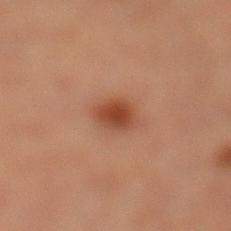The subject is a male aged around 60. This is a cross-polarized tile. A 15 mm close-up extracted from a 3D total-body photography capture. Automated tile analysis of the lesion measured a lesion–skin lightness drop of about 9 and a normalized lesion–skin contrast near 9. It also reported a border-irregularity index near 1.5/10, a within-lesion color-variation index near 2.5/10, and a peripheral color-asymmetry measure near 0.5. And it measured a classifier nevus-likeness of about 100/100 and a detector confidence of about 100 out of 100 that the crop contains a lesion. Longest diameter approximately 2.5 mm. From the leg.